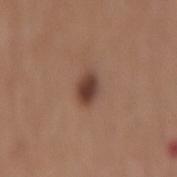- subject · male, roughly 75 years of age
- lesion diameter · about 3 mm
- anatomic site · the mid back
- automated metrics · a lesion color around L≈41 a*≈19 b*≈25 in CIELAB and about 13 CIELAB-L* units darker than the surrounding skin; a border-irregularity index near 1.5/10, internal color variation of about 3.5 on a 0–10 scale, and peripheral color asymmetry of about 1; a detector confidence of about 100 out of 100 that the crop contains a lesion
- image · 15 mm crop, total-body photography
- tile lighting · white-light illumination